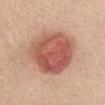workup=no biopsy performed (imaged during a skin exam)
patient=female, aged 48 to 52
illumination=white-light illumination
anatomic site=the abdomen
imaging modality=15 mm crop, total-body photography